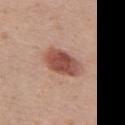{
  "biopsy_status": "not biopsied; imaged during a skin examination",
  "automated_metrics": {
    "area_mm2_approx": 11.0,
    "eccentricity": 0.8,
    "shape_asymmetry": 0.2,
    "vs_skin_darker_L": 15.0,
    "border_irregularity_0_10": 2.0,
    "color_variation_0_10": 4.5,
    "peripheral_color_asymmetry": 1.5
  },
  "patient": {
    "sex": "female",
    "age_approx": 40
  },
  "site": "chest",
  "lighting": "white-light",
  "image": {
    "source": "total-body photography crop",
    "field_of_view_mm": 15
  },
  "lesion_size": {
    "long_diameter_mm_approx": 5.0
  }
}